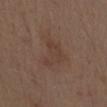<tbp_lesion>
<biopsy_status>not biopsied; imaged during a skin examination</biopsy_status>
<site>mid back</site>
<image>
  <source>total-body photography crop</source>
  <field_of_view_mm>15</field_of_view_mm>
</image>
<patient>
  <sex>male</sex>
  <age_approx>70</age_approx>
</patient>
</tbp_lesion>